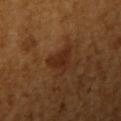The lesion was photographed on a routine skin check and not biopsied; there is no pathology result. The lesion is located on the left upper arm. A female patient, aged approximately 55. A 15 mm close-up extracted from a 3D total-body photography capture.